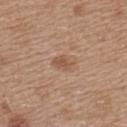{"biopsy_status": "not biopsied; imaged during a skin examination", "site": "left upper arm", "image": {"source": "total-body photography crop", "field_of_view_mm": 15}, "lesion_size": {"long_diameter_mm_approx": 2.5}, "patient": {"sex": "female", "age_approx": 40}, "lighting": "white-light", "automated_metrics": {"area_mm2_approx": 3.5, "eccentricity": 0.75, "shape_asymmetry": 0.25, "color_variation_0_10": 1.5, "peripheral_color_asymmetry": 0.5, "nevus_likeness_0_100": 0, "lesion_detection_confidence_0_100": 100}}